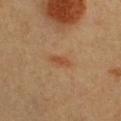{"biopsy_status": "not biopsied; imaged during a skin examination", "site": "chest", "lighting": "cross-polarized", "patient": {"sex": "female", "age_approx": 40}, "automated_metrics": {"cielab_L": 41, "cielab_a": 21, "cielab_b": 31, "vs_skin_darker_L": 7.0, "vs_skin_contrast_norm": 6.5}, "lesion_size": {"long_diameter_mm_approx": 2.5}, "image": {"source": "total-body photography crop", "field_of_view_mm": 15}}